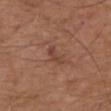diameter: ~3 mm (longest diameter)
site: the upper back
subject: male, aged 73–77
acquisition: ~15 mm crop, total-body skin-cancer survey